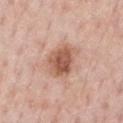Assessment: Recorded during total-body skin imaging; not selected for excision or biopsy. Context: On the chest. Cropped from a total-body skin-imaging series; the visible field is about 15 mm. The patient is a male in their 50s. The lesion's longest dimension is about 4 mm. Automated tile analysis of the lesion measured an area of roughly 10 mm². And it measured roughly 13 lightness units darker than nearby skin and a normalized border contrast of about 9. And it measured a within-lesion color-variation index near 4.5/10 and radial color variation of about 1.5. It also reported a lesion-detection confidence of about 100/100. The tile uses white-light illumination.